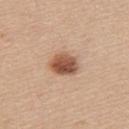Part of a total-body skin-imaging series; this lesion was reviewed on a skin check and was not flagged for biopsy.
This is a white-light tile.
On the upper back.
A 15 mm close-up extracted from a 3D total-body photography capture.
The subject is a female aged 43 to 47.